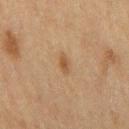Imaged during a routine full-body skin examination; the lesion was not biopsied and no histopathology is available.
A male subject in their mid- to late 70s.
The tile uses cross-polarized illumination.
Approximately 2.5 mm at its widest.
From the mid back.
A lesion tile, about 15 mm wide, cut from a 3D total-body photograph.